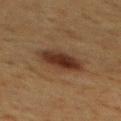Clinical impression:
Imaged during a routine full-body skin examination; the lesion was not biopsied and no histopathology is available.
Background:
Located on the mid back. A male subject aged around 60. The total-body-photography lesion software estimated a symmetry-axis asymmetry near 0.1. The analysis additionally found a lesion color around L≈28 a*≈16 b*≈24 in CIELAB, a lesion–skin lightness drop of about 10, and a lesion-to-skin contrast of about 10.5 (normalized; higher = more distinct). It also reported a border-irregularity rating of about 1.5/10 and a color-variation rating of about 4.5/10. The software also gave a lesion-detection confidence of about 100/100. A roughly 15 mm field-of-view crop from a total-body skin photograph.